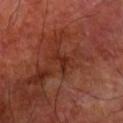Impression:
No biopsy was performed on this lesion — it was imaged during a full skin examination and was not determined to be concerning.
Acquisition and patient details:
Automated image analysis of the tile measured a shape eccentricity near 0.8 and a shape-asymmetry score of about 0.45 (0 = symmetric). The analysis additionally found a nevus-likeness score of about 0/100 and lesion-presence confidence of about 80/100. A male patient, aged around 70. A region of skin cropped from a whole-body photographic capture, roughly 15 mm wide. On the right forearm.